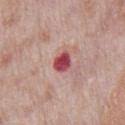| field | value |
|---|---|
| biopsy status | catalogued during a skin exam; not biopsied |
| anatomic site | the chest |
| image | ~15 mm crop, total-body skin-cancer survey |
| automated metrics | a mean CIELAB color near L≈48 a*≈35 b*≈20 and roughly 17 lightness units darker than nearby skin; internal color variation of about 3 on a 0–10 scale and peripheral color asymmetry of about 1; a detector confidence of about 100 out of 100 that the crop contains a lesion |
| lesion size | ≈2.5 mm |
| patient | male, approximately 70 years of age |